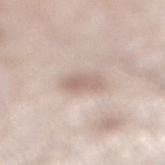* notes: no biopsy performed (imaged during a skin exam)
* image source: 15 mm crop, total-body photography
* patient: male, approximately 60 years of age
* lighting: white-light illumination
* body site: the right lower leg